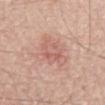This lesion was catalogued during total-body skin photography and was not selected for biopsy.
Cropped from a total-body skin-imaging series; the visible field is about 15 mm.
The lesion is on the mid back.
A male subject aged approximately 70.
The tile uses white-light illumination.